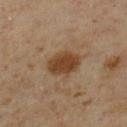follow-up=total-body-photography surveillance lesion; no biopsy | lesion diameter=~4 mm (longest diameter) | acquisition=~15 mm tile from a whole-body skin photo | illumination=cross-polarized illumination | anatomic site=the leg | patient=male, about 60 years old.